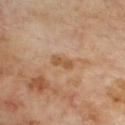Q: Illumination type?
A: cross-polarized illumination
Q: Where on the body is the lesion?
A: the front of the torso
Q: What is the lesion's diameter?
A: ~3 mm (longest diameter)
Q: What is the imaging modality?
A: ~15 mm tile from a whole-body skin photo
Q: Automated lesion metrics?
A: about 7 CIELAB-L* units darker than the surrounding skin and a normalized border contrast of about 6.5; a classifier nevus-likeness of about 0/100
Q: Patient demographics?
A: male, about 70 years old